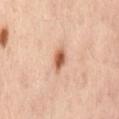<record>
<biopsy_status>not biopsied; imaged during a skin examination</biopsy_status>
</record>